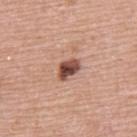Part of a total-body skin-imaging series; this lesion was reviewed on a skin check and was not flagged for biopsy.
A male patient approximately 70 years of age.
The tile uses white-light illumination.
The lesion's longest dimension is about 3 mm.
Located on the upper back.
This image is a 15 mm lesion crop taken from a total-body photograph.
An algorithmic analysis of the crop reported a footprint of about 5 mm², a shape eccentricity near 0.75, and a shape-asymmetry score of about 0.35 (0 = symmetric). And it measured a lesion color around L≈48 a*≈24 b*≈27 in CIELAB and a normalized lesion–skin contrast near 12.5. It also reported a nevus-likeness score of about 60/100 and a lesion-detection confidence of about 100/100.